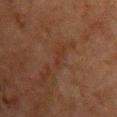Recorded during total-body skin imaging; not selected for excision or biopsy.
Located on the chest.
Cropped from a whole-body photographic skin survey; the tile spans about 15 mm.
Imaged with cross-polarized lighting.
The subject is a male aged 58 to 62.
An algorithmic analysis of the crop reported a lesion area of about 2 mm² and two-axis asymmetry of about 0.35. And it measured a lesion color around L≈25 a*≈17 b*≈23 in CIELAB and a lesion–skin lightness drop of about 4. It also reported an automated nevus-likeness rating near 0 out of 100.